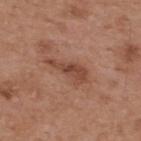- follow-up — imaged on a skin check; not biopsied
- imaging modality — ~15 mm crop, total-body skin-cancer survey
- location — the upper back
- automated metrics — a footprint of about 7.5 mm², an eccentricity of roughly 0.9, and two-axis asymmetry of about 0.45; an automated nevus-likeness rating near 15 out of 100
- tile lighting — white-light
- lesion diameter — ~5 mm (longest diameter)
- patient — male, in their mid- to late 50s